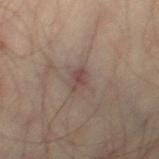notes = catalogued during a skin exam; not biopsied | diameter = ≈2.5 mm | image-analysis metrics = an area of roughly 4 mm² and an eccentricity of roughly 0.65; a border-irregularity index near 2/10, a color-variation rating of about 2/10, and radial color variation of about 0.5; lesion-presence confidence of about 100/100 | tile lighting = cross-polarized illumination | subject = male, aged around 35 | site = the right thigh | acquisition = ~15 mm crop, total-body skin-cancer survey.